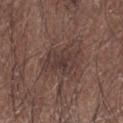follow-up = catalogued during a skin exam; not biopsied
patient = male, aged around 65
illumination = white-light illumination
location = the left upper arm
size = ~4 mm (longest diameter)
imaging modality = ~15 mm crop, total-body skin-cancer survey